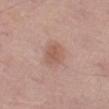No biopsy was performed on this lesion — it was imaged during a full skin examination and was not determined to be concerning.